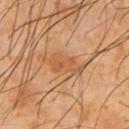Findings:
* workup — total-body-photography surveillance lesion; no biopsy
* illumination — cross-polarized
* patient — male, aged 63 to 67
* location — the arm
* TBP lesion metrics — a lesion area of about 9 mm², an eccentricity of roughly 0.9, and a symmetry-axis asymmetry near 0.3; roughly 6 lightness units darker than nearby skin and a normalized border contrast of about 5.5; border irregularity of about 5.5 on a 0–10 scale, a within-lesion color-variation index near 2.5/10, and a peripheral color-asymmetry measure near 1
* image — 15 mm crop, total-body photography